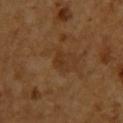Recorded during total-body skin imaging; not selected for excision or biopsy. Automated tile analysis of the lesion measured a footprint of about 3.5 mm², an eccentricity of roughly 0.8, and a shape-asymmetry score of about 0.4 (0 = symmetric). The analysis additionally found an automated nevus-likeness rating near 0 out of 100 and a lesion-detection confidence of about 100/100. From the upper back. A region of skin cropped from a whole-body photographic capture, roughly 15 mm wide. The patient is a female aged around 55.